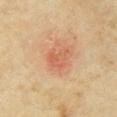<record>
<image>
  <source>total-body photography crop</source>
  <field_of_view_mm>15</field_of_view_mm>
</image>
<lesion_size>
  <long_diameter_mm_approx>3.0</long_diameter_mm_approx>
</lesion_size>
<site>right upper arm</site>
<lighting>cross-polarized</lighting>
<patient>
  <sex>female</sex>
  <age_approx>35</age_approx>
</patient>
</record>